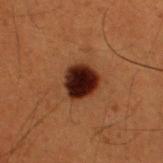Part of a total-body skin-imaging series; this lesion was reviewed on a skin check and was not flagged for biopsy.